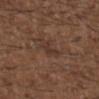Q: What is the anatomic site?
A: the back
Q: How large is the lesion?
A: about 3 mm
Q: Illumination type?
A: white-light illumination
Q: What is the imaging modality?
A: 15 mm crop, total-body photography
Q: What are the patient's age and sex?
A: male, aged approximately 50